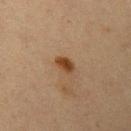The lesion was tiled from a total-body skin photograph and was not biopsied. A region of skin cropped from a whole-body photographic capture, roughly 15 mm wide. From the left upper arm. The subject is a female in their mid- to late 60s.